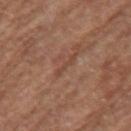Case summary:
- follow-up: imaged on a skin check; not biopsied
- body site: the left upper arm
- image: 15 mm crop, total-body photography
- subject: female, aged 78–82
- image-analysis metrics: a lesion area of about 2 mm², an eccentricity of roughly 0.95, and a symmetry-axis asymmetry near 0.35; an average lesion color of about L≈45 a*≈21 b*≈29 (CIELAB), roughly 6 lightness units darker than nearby skin, and a normalized lesion–skin contrast near 5; a border-irregularity index near 5/10
- lesion size: ≈3 mm
- illumination: white-light illumination